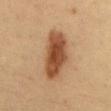Clinical impression:
Part of a total-body skin-imaging series; this lesion was reviewed on a skin check and was not flagged for biopsy.
Clinical summary:
Approximately 7 mm at its widest. From the back. The subject is a male aged approximately 35. A close-up tile cropped from a whole-body skin photograph, about 15 mm across. The tile uses cross-polarized illumination.